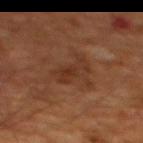Recorded during total-body skin imaging; not selected for excision or biopsy. On the chest. Longest diameter approximately 4.5 mm. Automated image analysis of the tile measured an average lesion color of about L≈29 a*≈19 b*≈27 (CIELAB), roughly 6 lightness units darker than nearby skin, and a normalized border contrast of about 6. The software also gave a border-irregularity index near 6/10, internal color variation of about 1.5 on a 0–10 scale, and peripheral color asymmetry of about 0.5. The analysis additionally found a detector confidence of about 100 out of 100 that the crop contains a lesion. A male patient aged approximately 60. Cropped from a total-body skin-imaging series; the visible field is about 15 mm. This is a cross-polarized tile.